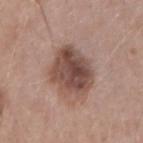Q: Was a biopsy performed?
A: imaged on a skin check; not biopsied
Q: Automated lesion metrics?
A: a lesion area of about 18 mm², an outline eccentricity of about 0.6 (0 = round, 1 = elongated), and a symmetry-axis asymmetry near 0.15; a border-irregularity index near 2/10 and peripheral color asymmetry of about 2.5; a detector confidence of about 100 out of 100 that the crop contains a lesion
Q: What is the lesion's diameter?
A: ≈5.5 mm
Q: How was this image acquired?
A: ~15 mm crop, total-body skin-cancer survey
Q: What lighting was used for the tile?
A: white-light illumination
Q: Where on the body is the lesion?
A: the left forearm
Q: What are the patient's age and sex?
A: female, roughly 45 years of age